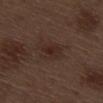No biopsy was performed on this lesion — it was imaged during a full skin examination and was not determined to be concerning. The patient is a male aged 68–72. A roughly 15 mm field-of-view crop from a total-body skin photograph. Imaged with white-light lighting. The lesion's longest dimension is about 2.5 mm. From the right thigh.